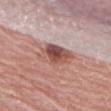The lesion is on the arm.
The tile uses white-light illumination.
The patient is a female aged around 65.
A 15 mm crop from a total-body photograph taken for skin-cancer surveillance.
An algorithmic analysis of the crop reported an area of roughly 11 mm², an outline eccentricity of about 0.85 (0 = round, 1 = elongated), and a symmetry-axis asymmetry near 0.25. The software also gave a mean CIELAB color near L≈53 a*≈24 b*≈25, roughly 12 lightness units darker than nearby skin, and a normalized lesion–skin contrast near 8.5.